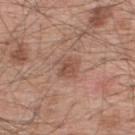{
  "image": {
    "source": "total-body photography crop",
    "field_of_view_mm": 15
  },
  "site": "upper back",
  "lighting": "white-light",
  "patient": {
    "sex": "male",
    "age_approx": 55
  },
  "lesion_size": {
    "long_diameter_mm_approx": 2.5
  }
}